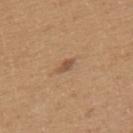  biopsy_status: not biopsied; imaged during a skin examination
  image:
    source: total-body photography crop
    field_of_view_mm: 15
  site: upper back
  automated_metrics:
    area_mm2_approx: 2.5
    eccentricity: 0.9
    shape_asymmetry: 0.25
    border_irregularity_0_10: 2.5
    color_variation_0_10: 0.0
    peripheral_color_asymmetry: 0.0
  lesion_size:
    long_diameter_mm_approx: 2.5
  patient:
    sex: male
    age_approx: 65